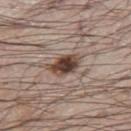Captured during whole-body skin photography for melanoma surveillance; the lesion was not biopsied.